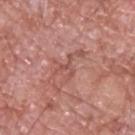– patient · male, about 60 years old
– acquisition · total-body-photography crop, ~15 mm field of view
– anatomic site · the upper back
– diameter · ≈3 mm
– TBP lesion metrics · an area of roughly 2.5 mm², an eccentricity of roughly 0.75, and a shape-asymmetry score of about 0.8 (0 = symmetric); an average lesion color of about L≈52 a*≈25 b*≈26 (CIELAB), a lesion–skin lightness drop of about 8, and a lesion-to-skin contrast of about 5.5 (normalized; higher = more distinct)
– tile lighting · white-light